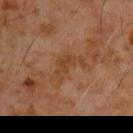Imaged during a routine full-body skin examination; the lesion was not biopsied and no histopathology is available. The lesion is located on the chest. Cropped from a total-body skin-imaging series; the visible field is about 15 mm. The lesion-visualizer software estimated a lesion area of about 8.5 mm², an eccentricity of roughly 0.85, and two-axis asymmetry of about 0.65. It also reported an average lesion color of about L≈40 a*≈20 b*≈32 (CIELAB). It also reported border irregularity of about 8 on a 0–10 scale, a within-lesion color-variation index near 2.5/10, and radial color variation of about 0.5. A male subject aged 58 to 62.